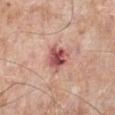Imaged during a routine full-body skin examination; the lesion was not biopsied and no histopathology is available. From the leg. A male patient, approximately 75 years of age. A 15 mm close-up tile from a total-body photography series done for melanoma screening.